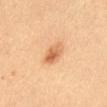Impression:
The lesion was photographed on a routine skin check and not biopsied; there is no pathology result.
Context:
The recorded lesion diameter is about 3.5 mm. Cropped from a whole-body photographic skin survey; the tile spans about 15 mm. The tile uses cross-polarized illumination. A female patient in their mid-30s. Located on the abdomen.